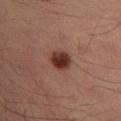Part of a total-body skin-imaging series; this lesion was reviewed on a skin check and was not flagged for biopsy. Automated image analysis of the tile measured an average lesion color of about L≈30 a*≈21 b*≈24 (CIELAB), about 13 CIELAB-L* units darker than the surrounding skin, and a normalized lesion–skin contrast near 12.5. The software also gave a classifier nevus-likeness of about 100/100 and a lesion-detection confidence of about 100/100. The lesion's longest dimension is about 3 mm. A male patient, aged around 35. Cropped from a whole-body photographic skin survey; the tile spans about 15 mm. From the left lower leg.